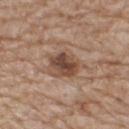notes: catalogued during a skin exam; not biopsied
acquisition: ~15 mm crop, total-body skin-cancer survey
subject: male, aged around 80
diameter: ≈5 mm
anatomic site: the upper back
automated lesion analysis: an average lesion color of about L≈48 a*≈18 b*≈27 (CIELAB) and a normalized border contrast of about 8.5; a border-irregularity index near 3/10 and a peripheral color-asymmetry measure near 2
tile lighting: white-light